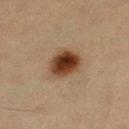No biopsy was performed on this lesion — it was imaged during a full skin examination and was not determined to be concerning. A roughly 15 mm field-of-view crop from a total-body skin photograph. Automated image analysis of the tile measured a lesion color around L≈36 a*≈17 b*≈28 in CIELAB. The analysis additionally found internal color variation of about 7.5 on a 0–10 scale and radial color variation of about 2. A female subject, roughly 40 years of age. From the right thigh. Imaged with cross-polarized lighting.